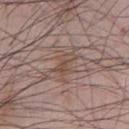follow-up: imaged on a skin check; not biopsied
site: the chest
image: ~15 mm tile from a whole-body skin photo
lighting: white-light
patient: male, aged around 65